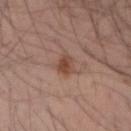{
  "biopsy_status": "not biopsied; imaged during a skin examination",
  "patient": {
    "sex": "male",
    "age_approx": 35
  },
  "lighting": "white-light",
  "site": "left forearm",
  "lesion_size": {
    "long_diameter_mm_approx": 3.0
  },
  "image": {
    "source": "total-body photography crop",
    "field_of_view_mm": 15
  },
  "automated_metrics": {
    "area_mm2_approx": 4.5,
    "eccentricity": 0.6,
    "shape_asymmetry": 0.3,
    "border_irregularity_0_10": 3.0,
    "color_variation_0_10": 3.0,
    "peripheral_color_asymmetry": 1.0,
    "nevus_likeness_0_100": 85,
    "lesion_detection_confidence_0_100": 100
  }
}